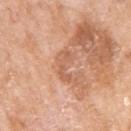biopsy_status: not biopsied; imaged during a skin examination
site: arm
image:
  source: total-body photography crop
  field_of_view_mm: 15
lighting: white-light
patient:
  sex: female
  age_approx: 75